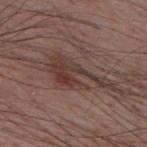The lesion was tiled from a total-body skin photograph and was not biopsied.